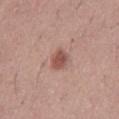Assessment:
Recorded during total-body skin imaging; not selected for excision or biopsy.
Acquisition and patient details:
A male subject in their mid-40s. Captured under white-light illumination. A 15 mm close-up extracted from a 3D total-body photography capture. The lesion is located on the abdomen. About 2.5 mm across.